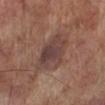{
  "biopsy_status": "not biopsied; imaged during a skin examination",
  "site": "left lower leg",
  "image": {
    "source": "total-body photography crop",
    "field_of_view_mm": 15
  },
  "lesion_size": {
    "long_diameter_mm_approx": 5.5
  },
  "patient": {
    "sex": "male",
    "age_approx": 70
  },
  "lighting": "white-light"
}